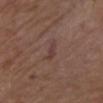| key | value |
|---|---|
| biopsy status | no biopsy performed (imaged during a skin exam) |
| site | the chest |
| patient | male, in their mid-70s |
| acquisition | ~15 mm crop, total-body skin-cancer survey |